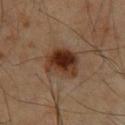patient: male, in their 60s | lighting: cross-polarized illumination | diameter: ≈4 mm | location: the chest | acquisition: ~15 mm crop, total-body skin-cancer survey.